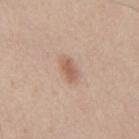Notes:
* biopsy status · no biopsy performed (imaged during a skin exam)
* tile lighting · white-light
* imaging modality · total-body-photography crop, ~15 mm field of view
* image-analysis metrics · an area of roughly 4 mm², an outline eccentricity of about 0.9 (0 = round, 1 = elongated), and two-axis asymmetry of about 0.25; a lesion-detection confidence of about 100/100
* patient · male, approximately 45 years of age
* location · the mid back